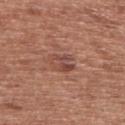Q: Was a biopsy performed?
A: imaged on a skin check; not biopsied
Q: Lesion location?
A: the upper back
Q: How was this image acquired?
A: 15 mm crop, total-body photography
Q: Patient demographics?
A: male, aged 53–57
Q: How large is the lesion?
A: ≈2.5 mm